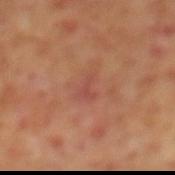Case summary:
– site · the mid back
– patient · male, aged approximately 70
– lesion diameter · about 3 mm
– acquisition · ~15 mm tile from a whole-body skin photo